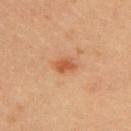Assessment: This lesion was catalogued during total-body skin photography and was not selected for biopsy. Image and clinical context: Captured under cross-polarized illumination. Cropped from a whole-body photographic skin survey; the tile spans about 15 mm. An algorithmic analysis of the crop reported border irregularity of about 2.5 on a 0–10 scale, a color-variation rating of about 2/10, and a peripheral color-asymmetry measure near 0.5. The software also gave a classifier nevus-likeness of about 65/100 and lesion-presence confidence of about 100/100. The lesion's longest dimension is about 2.5 mm. A female subject in their mid- to late 30s. The lesion is on the upper back.